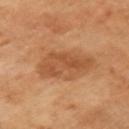Clinical impression: The lesion was photographed on a routine skin check and not biopsied; there is no pathology result. Context: Automated tile analysis of the lesion measured a shape-asymmetry score of about 0.3 (0 = symmetric). The analysis additionally found border irregularity of about 4 on a 0–10 scale, a color-variation rating of about 3.5/10, and radial color variation of about 1. A female patient aged 53–57. A lesion tile, about 15 mm wide, cut from a 3D total-body photograph. From the arm. The recorded lesion diameter is about 5.5 mm.